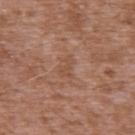Assessment: Captured during whole-body skin photography for melanoma surveillance; the lesion was not biopsied. Clinical summary: The recorded lesion diameter is about 2.5 mm. Cropped from a total-body skin-imaging series; the visible field is about 15 mm. The subject is a male approximately 45 years of age. The lesion is located on the upper back. The lesion-visualizer software estimated an outline eccentricity of about 0.8 (0 = round, 1 = elongated) and a shape-asymmetry score of about 0.45 (0 = symmetric). And it measured a border-irregularity index near 4.5/10, internal color variation of about 0.5 on a 0–10 scale, and radial color variation of about 0. Imaged with white-light lighting.